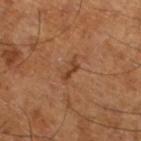The lesion is on the left lower leg.
Measured at roughly 3 mm in maximum diameter.
The tile uses cross-polarized illumination.
A male subject aged 63–67.
A roughly 15 mm field-of-view crop from a total-body skin photograph.